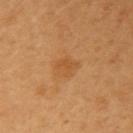Recorded during total-body skin imaging; not selected for excision or biopsy.
Automated image analysis of the tile measured an average lesion color of about L≈52 a*≈22 b*≈42 (CIELAB) and a normalized border contrast of about 5. The analysis additionally found a border-irregularity index near 2.5/10, a within-lesion color-variation index near 2/10, and a peripheral color-asymmetry measure near 1. The analysis additionally found a nevus-likeness score of about 10/100 and lesion-presence confidence of about 100/100.
On the arm.
A close-up tile cropped from a whole-body skin photograph, about 15 mm across.
A female patient, aged approximately 45.
About 3 mm across.
Imaged with cross-polarized lighting.